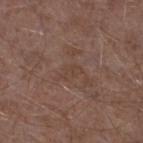No biopsy was performed on this lesion — it was imaged during a full skin examination and was not determined to be concerning.
Imaged with white-light lighting.
From the leg.
A lesion tile, about 15 mm wide, cut from a 3D total-body photograph.
A male patient, about 45 years old.
Longest diameter approximately 2.5 mm.